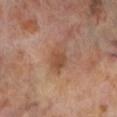{"biopsy_status": "not biopsied; imaged during a skin examination", "lighting": "cross-polarized", "image": {"source": "total-body photography crop", "field_of_view_mm": 15}, "site": "left lower leg", "patient": {"sex": "male", "age_approx": 70}, "lesion_size": {"long_diameter_mm_approx": 4.0}, "automated_metrics": {"border_irregularity_0_10": 2.5, "color_variation_0_10": 3.0, "peripheral_color_asymmetry": 1.0}}